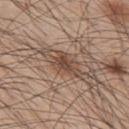Q: Was this lesion biopsied?
A: no biopsy performed (imaged during a skin exam)
Q: Patient demographics?
A: male, approximately 45 years of age
Q: Illumination type?
A: white-light
Q: What is the imaging modality?
A: ~15 mm crop, total-body skin-cancer survey
Q: Where on the body is the lesion?
A: the upper back